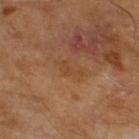{"image": {"source": "total-body photography crop", "field_of_view_mm": 15}, "lighting": "cross-polarized", "patient": {"sex": "male", "age_approx": 65}}